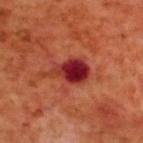Captured during whole-body skin photography for melanoma surveillance; the lesion was not biopsied. This image is a 15 mm lesion crop taken from a total-body photograph. About 5.5 mm across. The patient is a male roughly 70 years of age. The total-body-photography lesion software estimated an outline eccentricity of about 0.8 (0 = round, 1 = elongated). The software also gave a mean CIELAB color near L≈33 a*≈37 b*≈28, about 15 CIELAB-L* units darker than the surrounding skin, and a normalized border contrast of about 13. The software also gave a within-lesion color-variation index near 8.5/10. It also reported a classifier nevus-likeness of about 0/100 and a lesion-detection confidence of about 100/100. The lesion is located on the upper back. This is a cross-polarized tile.